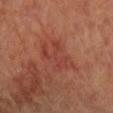follow-up: no biopsy performed (imaged during a skin exam); image source: ~15 mm tile from a whole-body skin photo; site: the left arm; patient: female, roughly 65 years of age; tile lighting: cross-polarized.